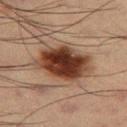follow-up: imaged on a skin check; not biopsied | diameter: ≈6 mm | body site: the right thigh | patient: male, in their mid-50s | automated lesion analysis: an area of roughly 22 mm²; a lesion color around L≈32 a*≈19 b*≈25 in CIELAB and a lesion–skin lightness drop of about 17; an automated nevus-likeness rating near 100 out of 100 and a lesion-detection confidence of about 100/100 | image: ~15 mm tile from a whole-body skin photo.